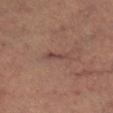Captured during whole-body skin photography for melanoma surveillance; the lesion was not biopsied.
This is a cross-polarized tile.
On the right lower leg.
A 15 mm close-up tile from a total-body photography series done for melanoma screening.
Longest diameter approximately 3 mm.
The subject is a female aged approximately 60.